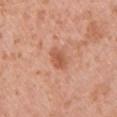follow-up — imaged on a skin check; not biopsied | subject — male, roughly 30 years of age | lighting — white-light | image — ~15 mm tile from a whole-body skin photo | TBP lesion metrics — a nevus-likeness score of about 10/100 and a lesion-detection confidence of about 100/100 | lesion diameter — about 3 mm | anatomic site — the front of the torso.